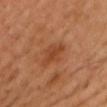workup: catalogued during a skin exam; not biopsied
location: the chest
size: ≈4.5 mm
lighting: cross-polarized illumination
automated metrics: a lesion area of about 6 mm² and an outline eccentricity of about 0.9 (0 = round, 1 = elongated); an automated nevus-likeness rating near 10 out of 100 and a lesion-detection confidence of about 100/100
patient: male, aged approximately 40
imaging modality: ~15 mm tile from a whole-body skin photo